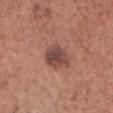Findings:
– follow-up · total-body-photography surveillance lesion; no biopsy
– imaging modality · total-body-photography crop, ~15 mm field of view
– lesion size · ~3.5 mm (longest diameter)
– subject · male, aged 63 to 67
– tile lighting · white-light illumination
– site · the head or neck
– automated lesion analysis · a border-irregularity rating of about 2.5/10, internal color variation of about 3.5 on a 0–10 scale, and a peripheral color-asymmetry measure near 1; a nevus-likeness score of about 35/100